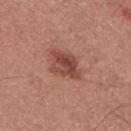<record>
  <biopsy_status>not biopsied; imaged during a skin examination</biopsy_status>
  <site>chest</site>
  <lighting>white-light</lighting>
  <patient>
    <sex>male</sex>
    <age_approx>35</age_approx>
  </patient>
  <image>
    <source>total-body photography crop</source>
    <field_of_view_mm>15</field_of_view_mm>
  </image>
  <lesion_size>
    <long_diameter_mm_approx>4.0</long_diameter_mm_approx>
  </lesion_size>
</record>